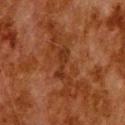Clinical impression:
No biopsy was performed on this lesion — it was imaged during a full skin examination and was not determined to be concerning.
Image and clinical context:
Cropped from a whole-body photographic skin survey; the tile spans about 15 mm. Imaged with cross-polarized lighting. The lesion is located on the upper back. Automated image analysis of the tile measured a lesion color around L≈25 a*≈21 b*≈28 in CIELAB, roughly 6 lightness units darker than nearby skin, and a normalized lesion–skin contrast near 6.5. The analysis additionally found a color-variation rating of about 1/10 and a peripheral color-asymmetry measure near 0.5. Longest diameter approximately 3.5 mm. A male subject, approximately 80 years of age.